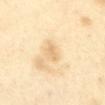body site = the abdomen | illumination = cross-polarized | patient = female, aged 53 to 57 | image source = ~15 mm crop, total-body skin-cancer survey | lesion diameter = about 2.5 mm.